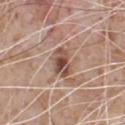Findings:
• workup · catalogued during a skin exam; not biopsied
• site · the chest
• subject · male, about 65 years old
• size · about 4 mm
• illumination · white-light
• acquisition · total-body-photography crop, ~15 mm field of view